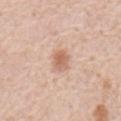No biopsy was performed on this lesion — it was imaged during a full skin examination and was not determined to be concerning.
A 15 mm close-up tile from a total-body photography series done for melanoma screening.
On the mid back.
The subject is a male aged 78 to 82.